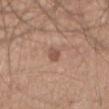Impression: No biopsy was performed on this lesion — it was imaged during a full skin examination and was not determined to be concerning. Background: The lesion is located on the abdomen. A 15 mm crop from a total-body photograph taken for skin-cancer surveillance. Longest diameter approximately 2.5 mm. A male patient, aged approximately 65.